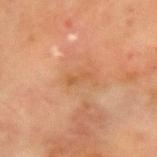This lesion was catalogued during total-body skin photography and was not selected for biopsy. The subject is a female aged 53 to 57. From the left forearm. A lesion tile, about 15 mm wide, cut from a 3D total-body photograph.